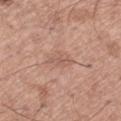{"biopsy_status": "not biopsied; imaged during a skin examination", "lesion_size": {"long_diameter_mm_approx": 3.0}, "automated_metrics": {"area_mm2_approx": 3.5, "eccentricity": 0.85, "shape_asymmetry": 0.4, "cielab_L": 57, "cielab_a": 21, "cielab_b": 27, "vs_skin_darker_L": 7.0, "vs_skin_contrast_norm": 5.0}, "image": {"source": "total-body photography crop", "field_of_view_mm": 15}, "patient": {"sex": "male", "age_approx": 65}, "site": "left thigh", "lighting": "white-light"}